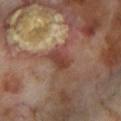notes: no biopsy performed (imaged during a skin exam); image source: 15 mm crop, total-body photography; subject: male, aged approximately 70; lighting: cross-polarized illumination; body site: the left lower leg.